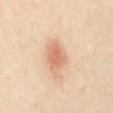Part of a total-body skin-imaging series; this lesion was reviewed on a skin check and was not flagged for biopsy.
A male subject, aged approximately 50.
A lesion tile, about 15 mm wide, cut from a 3D total-body photograph.
The lesion is on the abdomen.
The tile uses cross-polarized illumination.